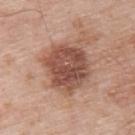workup = imaged on a skin check; not biopsied | image-analysis metrics = lesion-presence confidence of about 100/100 | lighting = white-light | patient = male, in their mid-50s | lesion diameter = about 5.5 mm | body site = the upper back | image source = 15 mm crop, total-body photography.